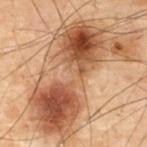biopsy_status: not biopsied; imaged during a skin examination
patient:
  sex: male
  age_approx: 70
lesion_size:
  long_diameter_mm_approx: 14.0
site: front of the torso
lighting: cross-polarized
image:
  source: total-body photography crop
  field_of_view_mm: 15